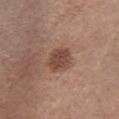The lesion was tiled from a total-body skin photograph and was not biopsied. On the chest. The patient is a female about 45 years old. Captured under white-light illumination. Measured at roughly 3.5 mm in maximum diameter. A 15 mm crop from a total-body photograph taken for skin-cancer surveillance.